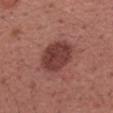{
  "biopsy_status": "not biopsied; imaged during a skin examination",
  "lesion_size": {
    "long_diameter_mm_approx": 5.0
  },
  "automated_metrics": {
    "area_mm2_approx": 15.0,
    "cielab_L": 40,
    "cielab_a": 25,
    "cielab_b": 23,
    "vs_skin_darker_L": 12.0,
    "vs_skin_contrast_norm": 9.5,
    "border_irregularity_0_10": 1.5,
    "color_variation_0_10": 3.0,
    "peripheral_color_asymmetry": 1.0,
    "nevus_likeness_0_100": 65,
    "lesion_detection_confidence_0_100": 100
  },
  "image": {
    "source": "total-body photography crop",
    "field_of_view_mm": 15
  },
  "site": "right forearm",
  "patient": {
    "sex": "female",
    "age_approx": 55
  }
}